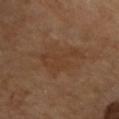No biopsy was performed on this lesion — it was imaged during a full skin examination and was not determined to be concerning.
Located on the chest.
A region of skin cropped from a whole-body photographic capture, roughly 15 mm wide.
Longest diameter approximately 5.5 mm.